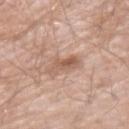Recorded during total-body skin imaging; not selected for excision or biopsy.
Captured under white-light illumination.
Located on the arm.
A close-up tile cropped from a whole-body skin photograph, about 15 mm across.
Longest diameter approximately 4 mm.
The subject is a male aged around 60.